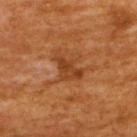biopsy status = imaged on a skin check; not biopsied
subject = male, roughly 60 years of age
automated lesion analysis = an area of roughly 7 mm² and two-axis asymmetry of about 0.4; a lesion color around L≈38 a*≈24 b*≈36 in CIELAB, roughly 8 lightness units darker than nearby skin, and a normalized lesion–skin contrast near 6.5; a nevus-likeness score of about 0/100 and a lesion-detection confidence of about 100/100
illumination = cross-polarized
diameter = ≈4 mm
site = the upper back
image = 15 mm crop, total-body photography